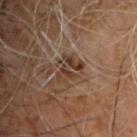<lesion>
  <image>
    <source>total-body photography crop</source>
    <field_of_view_mm>15</field_of_view_mm>
  </image>
  <automated_metrics>
    <area_mm2_approx>6.0</area_mm2_approx>
    <eccentricity>0.65</eccentricity>
    <shape_asymmetry>0.45</shape_asymmetry>
    <cielab_L>36</cielab_L>
    <cielab_a>16</cielab_a>
    <cielab_b>26</cielab_b>
    <vs_skin_darker_L>10.0</vs_skin_darker_L>
  </automated_metrics>
  <patient>
    <sex>male</sex>
    <age_approx>60</age_approx>
  </patient>
  <lighting>cross-polarized</lighting>
  <site>back</site>
  <lesion_size>
    <long_diameter_mm_approx>3.5</long_diameter_mm_approx>
  </lesion_size>
</lesion>